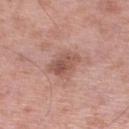Recorded during total-body skin imaging; not selected for excision or biopsy.
The subject is a male about 55 years old.
Located on the leg.
A 15 mm close-up tile from a total-body photography series done for melanoma screening.